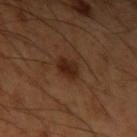Acquisition and patient details: On the left upper arm. A male patient in their mid- to late 60s. Measured at roughly 2.5 mm in maximum diameter. Captured under cross-polarized illumination. The lesion-visualizer software estimated border irregularity of about 1.5 on a 0–10 scale and peripheral color asymmetry of about 0.5. It also reported a nevus-likeness score of about 95/100 and a lesion-detection confidence of about 100/100. A 15 mm close-up tile from a total-body photography series done for melanoma screening.